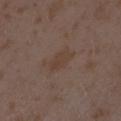Recorded during total-body skin imaging; not selected for excision or biopsy.
The recorded lesion diameter is about 3.5 mm.
Cropped from a total-body skin-imaging series; the visible field is about 15 mm.
A female patient, aged approximately 35.
The lesion is located on the left forearm.
Captured under white-light illumination.